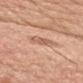Part of a total-body skin-imaging series; this lesion was reviewed on a skin check and was not flagged for biopsy.
A male subject, aged 53–57.
Automated image analysis of the tile measured a border-irregularity rating of about 3/10, a color-variation rating of about 3/10, and peripheral color asymmetry of about 1. And it measured an automated nevus-likeness rating near 5 out of 100 and a detector confidence of about 100 out of 100 that the crop contains a lesion.
A roughly 15 mm field-of-view crop from a total-body skin photograph.
About 3 mm across.
The lesion is located on the head or neck.